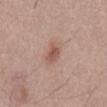Q: Is there a histopathology result?
A: no biopsy performed (imaged during a skin exam)
Q: Who is the patient?
A: male, aged approximately 55
Q: How was this image acquired?
A: 15 mm crop, total-body photography